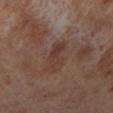Case summary:
– notes — imaged on a skin check; not biopsied
– patient — female, in their 50s
– acquisition — ~15 mm crop, total-body skin-cancer survey
– tile lighting — cross-polarized illumination
– lesion diameter — about 5 mm
– anatomic site — the left lower leg
– image-analysis metrics — a lesion color around L≈35 a*≈18 b*≈22 in CIELAB, about 6 CIELAB-L* units darker than the surrounding skin, and a normalized border contrast of about 5.5; border irregularity of about 3.5 on a 0–10 scale, internal color variation of about 3.5 on a 0–10 scale, and a peripheral color-asymmetry measure near 1.5